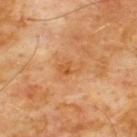No biopsy was performed on this lesion — it was imaged during a full skin examination and was not determined to be concerning. The recorded lesion diameter is about 3.5 mm. A roughly 15 mm field-of-view crop from a total-body skin photograph. Located on the chest. Captured under cross-polarized illumination. A male patient, in their 60s.